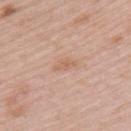Image and clinical context:
A female patient, approximately 65 years of age. Cropped from a total-body skin-imaging series; the visible field is about 15 mm. On the upper back. This is a white-light tile. Automated tile analysis of the lesion measured a footprint of about 2.5 mm² and a shape-asymmetry score of about 0.4 (0 = symmetric). It also reported a border-irregularity rating of about 4/10 and internal color variation of about 0 on a 0–10 scale. And it measured a lesion-detection confidence of about 100/100.